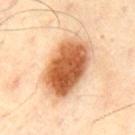Q: Was a biopsy performed?
A: catalogued during a skin exam; not biopsied
Q: What kind of image is this?
A: total-body-photography crop, ~15 mm field of view
Q: What is the anatomic site?
A: the front of the torso
Q: What lighting was used for the tile?
A: cross-polarized illumination
Q: What is the lesion's diameter?
A: ~7.5 mm (longest diameter)
Q: What are the patient's age and sex?
A: male, in their mid-60s